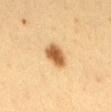workup: catalogued during a skin exam; not biopsied
image source: 15 mm crop, total-body photography
patient: female, about 30 years old
site: the mid back
illumination: cross-polarized
lesion diameter: about 3.5 mm